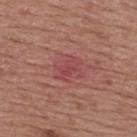Impression: Imaged during a routine full-body skin examination; the lesion was not biopsied and no histopathology is available. Context: A lesion tile, about 15 mm wide, cut from a 3D total-body photograph. The subject is a male roughly 55 years of age. The lesion-visualizer software estimated a lesion area of about 7 mm² and an outline eccentricity of about 0.6 (0 = round, 1 = elongated). The analysis additionally found an average lesion color of about L≈47 a*≈29 b*≈23 (CIELAB) and roughly 6 lightness units darker than nearby skin. The analysis additionally found a nevus-likeness score of about 0/100. The lesion is located on the upper back.